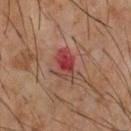| feature | finding |
|---|---|
| notes | total-body-photography surveillance lesion; no biopsy |
| diameter | about 3.5 mm |
| location | the chest |
| subject | male, aged 58–62 |
| illumination | cross-polarized illumination |
| image source | ~15 mm crop, total-body skin-cancer survey |